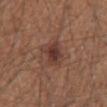image source = ~15 mm crop, total-body skin-cancer survey | diameter = ≈3.5 mm | anatomic site = the abdomen | automated metrics = a lesion area of about 8 mm², a shape eccentricity near 0.75, and two-axis asymmetry of about 0.15; a lesion–skin lightness drop of about 9 and a normalized lesion–skin contrast near 8; a border-irregularity rating of about 2/10, a color-variation rating of about 5.5/10, and peripheral color asymmetry of about 1.5 | subject = male, aged 63–67 | illumination = white-light.